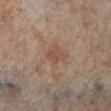* follow-up: imaged on a skin check; not biopsied
* imaging modality: ~15 mm tile from a whole-body skin photo
* patient: female, aged 48–52
* diameter: ~2.5 mm (longest diameter)
* anatomic site: the leg